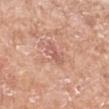{
  "biopsy_status": "not biopsied; imaged during a skin examination",
  "site": "left forearm",
  "patient": {
    "sex": "female",
    "age_approx": 75
  },
  "automated_metrics": {
    "area_mm2_approx": 3.5,
    "eccentricity": 0.9,
    "shape_asymmetry": 0.3,
    "cielab_L": 59,
    "cielab_a": 24,
    "cielab_b": 28,
    "vs_skin_darker_L": 8.0,
    "vs_skin_contrast_norm": 5.5,
    "border_irregularity_0_10": 4.0,
    "color_variation_0_10": 0.5,
    "peripheral_color_asymmetry": 0.0,
    "nevus_likeness_0_100": 0
  },
  "lesion_size": {
    "long_diameter_mm_approx": 3.5
  },
  "lighting": "white-light",
  "image": {
    "source": "total-body photography crop",
    "field_of_view_mm": 15
  }
}